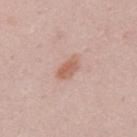biopsy status: total-body-photography surveillance lesion; no biopsy
subject: male, in their mid-20s
lighting: white-light illumination
image source: 15 mm crop, total-body photography
body site: the mid back